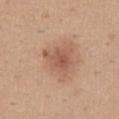The lesion was tiled from a total-body skin photograph and was not biopsied.
Captured under white-light illumination.
Located on the mid back.
A close-up tile cropped from a whole-body skin photograph, about 15 mm across.
A female patient, approximately 30 years of age.